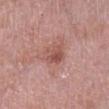This lesion was catalogued during total-body skin photography and was not selected for biopsy. On the right lower leg. The lesion-visualizer software estimated a lesion–skin lightness drop of about 9 and a normalized lesion–skin contrast near 6.5. The analysis additionally found peripheral color asymmetry of about 2. It also reported a classifier nevus-likeness of about 0/100. About 3 mm across. A male patient, in their mid- to late 70s. A region of skin cropped from a whole-body photographic capture, roughly 15 mm wide. The tile uses white-light illumination.